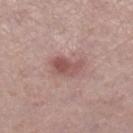Findings:
• biopsy status — imaged on a skin check; not biopsied
• anatomic site — the right lower leg
• lighting — white-light illumination
• subject — female, aged 63 to 67
• image — ~15 mm tile from a whole-body skin photo